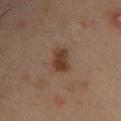<case>
  <biopsy_status>not biopsied; imaged during a skin examination</biopsy_status>
  <image>
    <source>total-body photography crop</source>
    <field_of_view_mm>15</field_of_view_mm>
  </image>
  <site>right upper arm</site>
  <lighting>cross-polarized</lighting>
  <patient>
    <sex>male</sex>
    <age_approx>40</age_approx>
  </patient>
  <lesion_size>
    <long_diameter_mm_approx>3.5</long_diameter_mm_approx>
  </lesion_size>
</case>